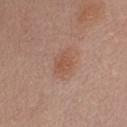biopsy status: catalogued during a skin exam; not biopsied.